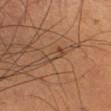Clinical impression: The lesion was tiled from a total-body skin photograph and was not biopsied. Acquisition and patient details: The patient is a male aged around 60. Automated image analysis of the tile measured a mean CIELAB color near L≈44 a*≈19 b*≈31, about 6 CIELAB-L* units darker than the surrounding skin, and a lesion-to-skin contrast of about 5.5 (normalized; higher = more distinct). It also reported a border-irregularity rating of about 4/10, internal color variation of about 0 on a 0–10 scale, and radial color variation of about 0. On the left lower leg. A lesion tile, about 15 mm wide, cut from a 3D total-body photograph. About 3.5 mm across.